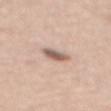No biopsy was performed on this lesion — it was imaged during a full skin examination and was not determined to be concerning.
Cropped from a total-body skin-imaging series; the visible field is about 15 mm.
The subject is a male approximately 60 years of age.
The lesion is on the upper back.
This is a white-light tile.
About 3 mm across.